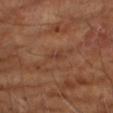* notes — catalogued during a skin exam; not biopsied
* location — the arm
* imaging modality — total-body-photography crop, ~15 mm field of view
* patient — male, about 65 years old